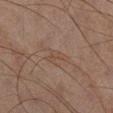{"biopsy_status": "not biopsied; imaged during a skin examination", "patient": {"sex": "male", "age_approx": 45}, "image": {"source": "total-body photography crop", "field_of_view_mm": 15}, "lesion_size": {"long_diameter_mm_approx": 3.0}, "site": "right lower leg", "lighting": "cross-polarized"}